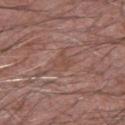Captured during whole-body skin photography for melanoma surveillance; the lesion was not biopsied.
The lesion is on the arm.
Automated tile analysis of the lesion measured an outline eccentricity of about 0.6 (0 = round, 1 = elongated) and two-axis asymmetry of about 0.35. And it measured a lesion color around L≈48 a*≈20 b*≈25 in CIELAB and about 5 CIELAB-L* units darker than the surrounding skin. The analysis additionally found a border-irregularity rating of about 4/10, internal color variation of about 2 on a 0–10 scale, and peripheral color asymmetry of about 0.5.
The tile uses white-light illumination.
A lesion tile, about 15 mm wide, cut from a 3D total-body photograph.
The recorded lesion diameter is about 3 mm.
A male patient, aged 48 to 52.